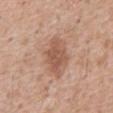On the abdomen. Longest diameter approximately 5.5 mm. The patient is a male aged approximately 60. A roughly 15 mm field-of-view crop from a total-body skin photograph. Captured under white-light illumination.